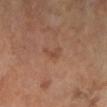follow-up: no biopsy performed (imaged during a skin exam)
anatomic site: the left leg
subject: male, aged 63–67
image: ~15 mm crop, total-body skin-cancer survey
lesion size: ≈2.5 mm
image-analysis metrics: a nevus-likeness score of about 10/100 and lesion-presence confidence of about 100/100